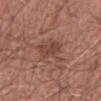This lesion was catalogued during total-body skin photography and was not selected for biopsy. This image is a 15 mm lesion crop taken from a total-body photograph. A male subject, in their 40s. The lesion-visualizer software estimated an area of roughly 9 mm², a shape eccentricity near 0.8, and a shape-asymmetry score of about 0.45 (0 = symmetric). The software also gave a mean CIELAB color near L≈45 a*≈22 b*≈25 and a lesion-to-skin contrast of about 6.5 (normalized; higher = more distinct). And it measured a color-variation rating of about 2.5/10 and radial color variation of about 1. The software also gave an automated nevus-likeness rating near 5 out of 100 and a detector confidence of about 100 out of 100 that the crop contains a lesion. The tile uses white-light illumination. The recorded lesion diameter is about 5 mm. The lesion is located on the right upper arm.